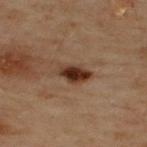Part of a total-body skin-imaging series; this lesion was reviewed on a skin check and was not flagged for biopsy. From the upper back. A region of skin cropped from a whole-body photographic capture, roughly 15 mm wide. The subject is a male aged 48 to 52. This is a cross-polarized tile. The lesion's longest dimension is about 4 mm.